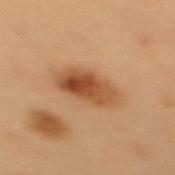Assessment: Part of a total-body skin-imaging series; this lesion was reviewed on a skin check and was not flagged for biopsy.